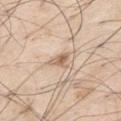<record>
  <biopsy_status>not biopsied; imaged during a skin examination</biopsy_status>
  <lesion_size>
    <long_diameter_mm_approx>2.5</long_diameter_mm_approx>
  </lesion_size>
  <lighting>white-light</lighting>
  <patient>
    <sex>male</sex>
    <age_approx>70</age_approx>
  </patient>
  <image>
    <source>total-body photography crop</source>
    <field_of_view_mm>15</field_of_view_mm>
  </image>
  <site>right thigh</site>
</record>